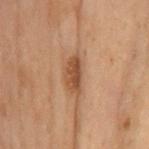<case>
<biopsy_status>not biopsied; imaged during a skin examination</biopsy_status>
<patient>
  <sex>male</sex>
  <age_approx>70</age_approx>
</patient>
<image>
  <source>total-body photography crop</source>
  <field_of_view_mm>15</field_of_view_mm>
</image>
<lighting>cross-polarized</lighting>
<site>chest</site>
</case>